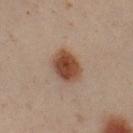Imaged during a routine full-body skin examination; the lesion was not biopsied and no histopathology is available.
The subject is a male aged 48 to 52.
On the left forearm.
Automated image analysis of the tile measured an area of roughly 10 mm², a shape eccentricity near 0.6, and a shape-asymmetry score of about 0.1 (0 = symmetric). And it measured roughly 13 lightness units darker than nearby skin and a normalized lesion–skin contrast near 11.
This is a cross-polarized tile.
A close-up tile cropped from a whole-body skin photograph, about 15 mm across.